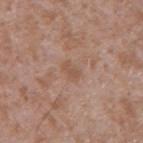Clinical impression: No biopsy was performed on this lesion — it was imaged during a full skin examination and was not determined to be concerning. Context: Imaged with white-light lighting. On the arm. A 15 mm close-up extracted from a 3D total-body photography capture. The subject is a male roughly 45 years of age.